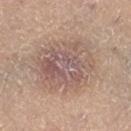Assessment:
Part of a total-body skin-imaging series; this lesion was reviewed on a skin check and was not flagged for biopsy.
Background:
Located on the right lower leg. A female patient aged 58–62. A 15 mm close-up tile from a total-body photography series done for melanoma screening. An algorithmic analysis of the crop reported a lesion color around L≈56 a*≈17 b*≈23 in CIELAB and roughly 9 lightness units darker than nearby skin. The tile uses white-light illumination. The lesion's longest dimension is about 7 mm.